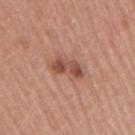{
  "biopsy_status": "not biopsied; imaged during a skin examination",
  "image": {
    "source": "total-body photography crop",
    "field_of_view_mm": 15
  },
  "site": "right upper arm",
  "automated_metrics": {
    "eccentricity": 0.9,
    "shape_asymmetry": 0.35,
    "vs_skin_darker_L": 12.0
  },
  "lighting": "white-light",
  "patient": {
    "sex": "male",
    "age_approx": 55
  }
}